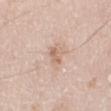Q: Is there a histopathology result?
A: catalogued during a skin exam; not biopsied
Q: Illumination type?
A: white-light
Q: What kind of image is this?
A: 15 mm crop, total-body photography
Q: Lesion size?
A: ~2.5 mm (longest diameter)
Q: What are the patient's age and sex?
A: male, roughly 60 years of age
Q: Lesion location?
A: the leg